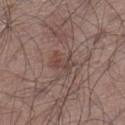biopsy_status: not biopsied; imaged during a skin examination
image:
  source: total-body photography crop
  field_of_view_mm: 15
patient:
  sex: male
  age_approx: 65
site: left lower leg
lesion_size:
  long_diameter_mm_approx: 3.0
lighting: white-light